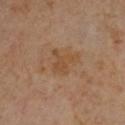Q: Is there a histopathology result?
A: no biopsy performed (imaged during a skin exam)
Q: Patient demographics?
A: female
Q: What is the imaging modality?
A: 15 mm crop, total-body photography
Q: What is the anatomic site?
A: the chest